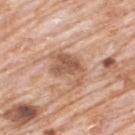The lesion was photographed on a routine skin check and not biopsied; there is no pathology result. Located on the upper back. The patient is a male in their 80s. An algorithmic analysis of the crop reported a border-irregularity index near 5/10 and a color-variation rating of about 4/10. A close-up tile cropped from a whole-body skin photograph, about 15 mm across. Imaged with white-light lighting.